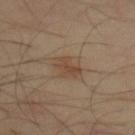Assessment: The lesion was photographed on a routine skin check and not biopsied; there is no pathology result. Background: A region of skin cropped from a whole-body photographic capture, roughly 15 mm wide. The lesion is on the front of the torso. Approximately 3 mm at its widest. The subject is about 65 years old.